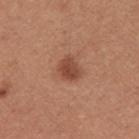workup=catalogued during a skin exam; not biopsied
image=~15 mm tile from a whole-body skin photo
patient=female, in their mid- to late 20s
site=the right upper arm